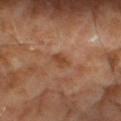Context:
Longest diameter approximately 3 mm. A male patient about 65 years old. A 15 mm close-up extracted from a 3D total-body photography capture.